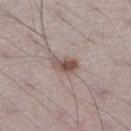notes: total-body-photography surveillance lesion; no biopsy | acquisition: ~15 mm tile from a whole-body skin photo | tile lighting: white-light illumination | subject: male, aged around 70 | size: ~3 mm (longest diameter) | location: the right lower leg | automated lesion analysis: about 12 CIELAB-L* units darker than the surrounding skin and a normalized border contrast of about 8.5; a border-irregularity rating of about 3/10, a within-lesion color-variation index near 4.5/10, and a peripheral color-asymmetry measure near 1.5; a nevus-likeness score of about 95/100 and a lesion-detection confidence of about 100/100.